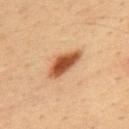Q: Was a biopsy performed?
A: no biopsy performed (imaged during a skin exam)
Q: How was this image acquired?
A: 15 mm crop, total-body photography
Q: What lighting was used for the tile?
A: cross-polarized
Q: Lesion size?
A: ~5 mm (longest diameter)
Q: Who is the patient?
A: male, aged around 35
Q: What did automated image analysis measure?
A: a footprint of about 8.5 mm², an eccentricity of roughly 0.9, and a shape-asymmetry score of about 0.25 (0 = symmetric); border irregularity of about 3 on a 0–10 scale, a color-variation rating of about 4.5/10, and radial color variation of about 1; a classifier nevus-likeness of about 100/100 and lesion-presence confidence of about 100/100
Q: Where on the body is the lesion?
A: the upper back